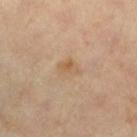Imaged during a routine full-body skin examination; the lesion was not biopsied and no histopathology is available.
The lesion is located on the left lower leg.
A lesion tile, about 15 mm wide, cut from a 3D total-body photograph.
A subject about 60 years old.